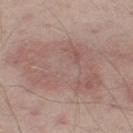Notes:
- follow-up · imaged on a skin check; not biopsied
- lighting · white-light illumination
- site · the right thigh
- TBP lesion metrics · a nevus-likeness score of about 0/100 and a lesion-detection confidence of about 80/100
- size · ≈15 mm
- imaging modality · ~15 mm crop, total-body skin-cancer survey
- patient · male, about 65 years old